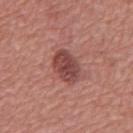biopsy_status: not biopsied; imaged during a skin examination
image:
  source: total-body photography crop
  field_of_view_mm: 15
patient:
  sex: male
  age_approx: 65
lesion_size:
  long_diameter_mm_approx: 4.0
site: mid back
automated_metrics:
  shape_asymmetry: 0.1
  cielab_L: 45
  cielab_a: 26
  cielab_b: 23
  vs_skin_darker_L: 12.0
  border_irregularity_0_10: 1.5
  color_variation_0_10: 3.0
  peripheral_color_asymmetry: 1.0
lighting: white-light